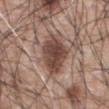Imaged during a routine full-body skin examination; the lesion was not biopsied and no histopathology is available.
Imaged with white-light lighting.
A male subject aged 58–62.
Approximately 5 mm at its widest.
A 15 mm close-up extracted from a 3D total-body photography capture.
Located on the abdomen.